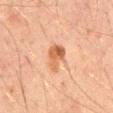<case>
  <biopsy_status>not biopsied; imaged during a skin examination</biopsy_status>
  <lesion_size>
    <long_diameter_mm_approx>4.0</long_diameter_mm_approx>
  </lesion_size>
  <site>chest</site>
  <patient>
    <sex>male</sex>
    <age_approx>45</age_approx>
  </patient>
  <image>
    <source>total-body photography crop</source>
    <field_of_view_mm>15</field_of_view_mm>
  </image>
</case>